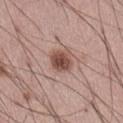No biopsy was performed on this lesion — it was imaged during a full skin examination and was not determined to be concerning. Imaged with white-light lighting. About 3 mm across. The total-body-photography lesion software estimated a nevus-likeness score of about 95/100. The patient is a male aged 53 to 57. On the abdomen. A lesion tile, about 15 mm wide, cut from a 3D total-body photograph.